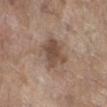The lesion was photographed on a routine skin check and not biopsied; there is no pathology result. Approximately 4.5 mm at its widest. From the leg. A region of skin cropped from a whole-body photographic capture, roughly 15 mm wide. The patient is a female aged approximately 85 or older. Captured under white-light illumination.